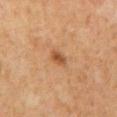Clinical impression: The lesion was photographed on a routine skin check and not biopsied; there is no pathology result. Context: This is a cross-polarized tile. An algorithmic analysis of the crop reported a footprint of about 3.5 mm², an outline eccentricity of about 0.7 (0 = round, 1 = elongated), and a symmetry-axis asymmetry near 0.25. Approximately 2.5 mm at its widest. A 15 mm close-up extracted from a 3D total-body photography capture. A male subject aged 58 to 62. The lesion is on the back.